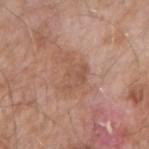Assessment:
Imaged during a routine full-body skin examination; the lesion was not biopsied and no histopathology is available.
Context:
A roughly 15 mm field-of-view crop from a total-body skin photograph. The lesion is on the arm. The tile uses white-light illumination. A male subject, in their mid-70s. The recorded lesion diameter is about 4 mm. Automated tile analysis of the lesion measured an area of roughly 7 mm² and two-axis asymmetry of about 0.5. It also reported an automated nevus-likeness rating near 0 out of 100 and lesion-presence confidence of about 100/100.